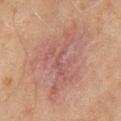biopsy_status: not biopsied; imaged during a skin examination
lighting: cross-polarized
lesion_size:
  long_diameter_mm_approx: 9.5
patient:
  sex: male
  age_approx: 45
automated_metrics:
  area_mm2_approx: 50.0
  eccentricity: 0.7
  shape_asymmetry: 0.25
  border_irregularity_0_10: 4.0
  color_variation_0_10: 5.0
  peripheral_color_asymmetry: 1.5
  nevus_likeness_0_100: 0
  lesion_detection_confidence_0_100: 100
site: mid back
image:
  source: total-body photography crop
  field_of_view_mm: 15